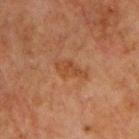Assessment: Imaged during a routine full-body skin examination; the lesion was not biopsied and no histopathology is available. Image and clinical context: From the chest. A 15 mm close-up tile from a total-body photography series done for melanoma screening. Approximately 3.5 mm at its widest. A male subject aged 63 to 67. Imaged with cross-polarized lighting.